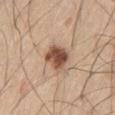A male subject in their 60s.
Cropped from a total-body skin-imaging series; the visible field is about 15 mm.
The total-body-photography lesion software estimated an eccentricity of roughly 0.6 and two-axis asymmetry of about 0.25. It also reported a border-irregularity rating of about 2.5/10, internal color variation of about 5 on a 0–10 scale, and radial color variation of about 1.5. The software also gave a detector confidence of about 100 out of 100 that the crop contains a lesion.
On the left thigh.